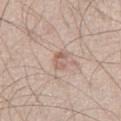Clinical impression:
The lesion was photographed on a routine skin check and not biopsied; there is no pathology result.